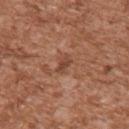notes = total-body-photography surveillance lesion; no biopsy
location = the arm
diameter = about 2.5 mm
lighting = white-light illumination
subject = male, in their mid-40s
acquisition = total-body-photography crop, ~15 mm field of view